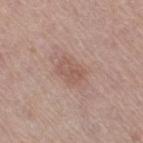| key | value |
|---|---|
| notes | no biopsy performed (imaged during a skin exam) |
| body site | the left thigh |
| illumination | white-light |
| patient | female, roughly 65 years of age |
| image source | total-body-photography crop, ~15 mm field of view |
| automated metrics | a mean CIELAB color near L≈55 a*≈20 b*≈25, about 7 CIELAB-L* units darker than the surrounding skin, and a normalized lesion–skin contrast near 5; a border-irregularity rating of about 3/10, a within-lesion color-variation index near 3.5/10, and peripheral color asymmetry of about 1.5; a nevus-likeness score of about 10/100 and a detector confidence of about 100 out of 100 that the crop contains a lesion |